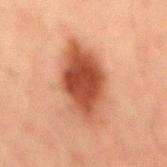{
  "biopsy_status": "not biopsied; imaged during a skin examination",
  "image": {
    "source": "total-body photography crop",
    "field_of_view_mm": 15
  },
  "site": "mid back",
  "patient": {
    "sex": "male",
    "age_approx": 50
  },
  "lesion_size": {
    "long_diameter_mm_approx": 8.0
  }
}